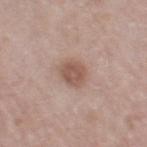Imaged during a routine full-body skin examination; the lesion was not biopsied and no histopathology is available. The lesion is on the right thigh. Approximately 3 mm at its widest. A female subject, aged 58–62. The lesion-visualizer software estimated an outline eccentricity of about 0.45 (0 = round, 1 = elongated). Imaged with white-light lighting. This image is a 15 mm lesion crop taken from a total-body photograph.